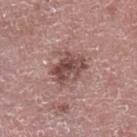<record>
  <biopsy_status>not biopsied; imaged during a skin examination</biopsy_status>
  <site>left lower leg</site>
  <patient>
    <sex>male</sex>
    <age_approx>75</age_approx>
  </patient>
  <image>
    <source>total-body photography crop</source>
    <field_of_view_mm>15</field_of_view_mm>
  </image>
  <lighting>white-light</lighting>
</record>